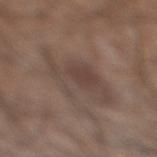Impression: Part of a total-body skin-imaging series; this lesion was reviewed on a skin check and was not flagged for biopsy. Image and clinical context: The subject is a male in their mid-50s. On the right lower leg. The lesion's longest dimension is about 7 mm. A close-up tile cropped from a whole-body skin photograph, about 15 mm across. Imaged with white-light lighting. Automated tile analysis of the lesion measured a lesion area of about 18 mm², an outline eccentricity of about 0.85 (0 = round, 1 = elongated), and two-axis asymmetry of about 0.45. It also reported roughly 7 lightness units darker than nearby skin and a normalized lesion–skin contrast near 6. And it measured border irregularity of about 6 on a 0–10 scale and a color-variation rating of about 3.5/10. The analysis additionally found a detector confidence of about 95 out of 100 that the crop contains a lesion.